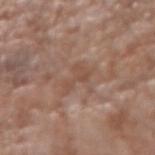biopsy_status: not biopsied; imaged during a skin examination
site: arm
patient:
  sex: male
  age_approx: 75
lighting: white-light
automated_metrics:
  area_mm2_approx: 5.0
  eccentricity: 0.85
  shape_asymmetry: 0.5
  cielab_L: 49
  cielab_a: 19
  cielab_b: 27
  vs_skin_contrast_norm: 5.0
  border_irregularity_0_10: 6.0
  color_variation_0_10: 1.5
  peripheral_color_asymmetry: 0.5
image:
  source: total-body photography crop
  field_of_view_mm: 15
lesion_size:
  long_diameter_mm_approx: 4.0